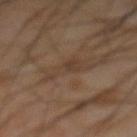follow-up: imaged on a skin check; not biopsied
imaging modality: ~15 mm crop, total-body skin-cancer survey
subject: male, roughly 65 years of age
TBP lesion metrics: a footprint of about 7.5 mm², an eccentricity of roughly 0.85, and a shape-asymmetry score of about 0.45 (0 = symmetric); a lesion color around L≈36 a*≈14 b*≈25 in CIELAB and about 5 CIELAB-L* units darker than the surrounding skin; peripheral color asymmetry of about 0.5
body site: the front of the torso
lesion size: ≈4.5 mm